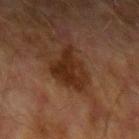follow-up = catalogued during a skin exam; not biopsied | acquisition = 15 mm crop, total-body photography | subject = male, aged 73–77 | automated lesion analysis = a border-irregularity index near 4/10, a within-lesion color-variation index near 3.5/10, and a peripheral color-asymmetry measure near 1.5 | size = ≈4.5 mm | body site = the left upper arm.